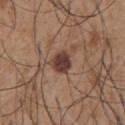{"biopsy_status": "not biopsied; imaged during a skin examination", "site": "chest", "lesion_size": {"long_diameter_mm_approx": 2.5}, "patient": {"sex": "male", "age_approx": 55}, "image": {"source": "total-body photography crop", "field_of_view_mm": 15}}